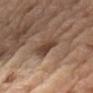notes: no biopsy performed (imaged during a skin exam)
size: ≈4 mm
tile lighting: white-light illumination
anatomic site: the left upper arm
subject: male, aged around 70
automated metrics: an area of roughly 7.5 mm², an eccentricity of roughly 0.85, and a shape-asymmetry score of about 0.3 (0 = symmetric); border irregularity of about 3.5 on a 0–10 scale and a peripheral color-asymmetry measure near 1; a classifier nevus-likeness of about 55/100 and lesion-presence confidence of about 95/100
image source: ~15 mm crop, total-body skin-cancer survey